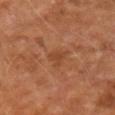{
  "patient": {
    "sex": "male",
    "age_approx": 55
  },
  "image": {
    "source": "total-body photography crop",
    "field_of_view_mm": 15
  },
  "lighting": "cross-polarized",
  "site": "right forearm",
  "automated_metrics": {
    "vs_skin_darker_L": 6.0,
    "vs_skin_contrast_norm": 5.0,
    "nevus_likeness_0_100": 0,
    "lesion_detection_confidence_0_100": 100
  },
  "lesion_size": {
    "long_diameter_mm_approx": 3.0
  }
}